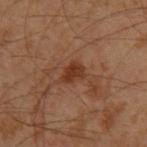Part of a total-body skin-imaging series; this lesion was reviewed on a skin check and was not flagged for biopsy.
Measured at roughly 3 mm in maximum diameter.
The subject is a male aged 28 to 32.
The lesion-visualizer software estimated a footprint of about 4.5 mm² and an eccentricity of roughly 0.7. It also reported a mean CIELAB color near L≈35 a*≈23 b*≈31, about 9 CIELAB-L* units darker than the surrounding skin, and a normalized border contrast of about 8.5. And it measured border irregularity of about 2.5 on a 0–10 scale and peripheral color asymmetry of about 0.5. It also reported an automated nevus-likeness rating near 65 out of 100.
From the left upper arm.
A roughly 15 mm field-of-view crop from a total-body skin photograph.